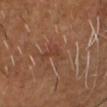Impression: Imaged during a routine full-body skin examination; the lesion was not biopsied and no histopathology is available. Acquisition and patient details: A male patient, approximately 60 years of age. About 3 mm across. The lesion is located on the head or neck. A 15 mm crop from a total-body photograph taken for skin-cancer surveillance. The tile uses cross-polarized illumination.